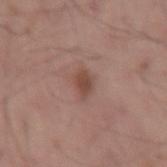– notes: catalogued during a skin exam; not biopsied
– body site: the right thigh
– lesion size: ≈3 mm
– tile lighting: white-light illumination
– patient: male, aged approximately 55
– imaging modality: ~15 mm tile from a whole-body skin photo